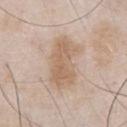Part of a total-body skin-imaging series; this lesion was reviewed on a skin check and was not flagged for biopsy. Cropped from a total-body skin-imaging series; the visible field is about 15 mm. The patient is a male aged around 55. From the chest. The lesion's longest dimension is about 6.5 mm. Imaged with white-light lighting. The total-body-photography lesion software estimated a footprint of about 17 mm². The software also gave an average lesion color of about L≈62 a*≈16 b*≈30 (CIELAB) and a lesion–skin lightness drop of about 9.